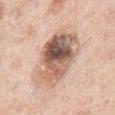No biopsy was performed on this lesion — it was imaged during a full skin examination and was not determined to be concerning. A male patient, aged 73 to 77. The lesion is on the chest. Cropped from a whole-body photographic skin survey; the tile spans about 15 mm.